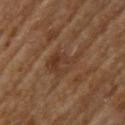Findings:
* workup: no biopsy performed (imaged during a skin exam)
* size: about 4.5 mm
* subject: male, in their mid- to late 60s
* tile lighting: cross-polarized illumination
* TBP lesion metrics: a footprint of about 9.5 mm², an eccentricity of roughly 0.7, and a shape-asymmetry score of about 0.45 (0 = symmetric); a lesion color around L≈32 a*≈17 b*≈25 in CIELAB, a lesion–skin lightness drop of about 6, and a normalized border contrast of about 6; a border-irregularity index near 5.5/10 and a within-lesion color-variation index near 4/10
* site: the upper back
* acquisition: 15 mm crop, total-body photography